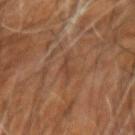Part of a total-body skin-imaging series; this lesion was reviewed on a skin check and was not flagged for biopsy.
Automated image analysis of the tile measured border irregularity of about 7 on a 0–10 scale. And it measured an automated nevus-likeness rating near 0 out of 100 and lesion-presence confidence of about 90/100.
A male subject aged 58–62.
Imaged with cross-polarized lighting.
Located on the right upper arm.
A roughly 15 mm field-of-view crop from a total-body skin photograph.